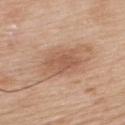This lesion was catalogued during total-body skin photography and was not selected for biopsy.
Automated tile analysis of the lesion measured a lesion area of about 9.5 mm², a shape eccentricity near 0.7, and a shape-asymmetry score of about 0.2 (0 = symmetric). The analysis additionally found a lesion color around L≈56 a*≈21 b*≈31 in CIELAB, roughly 9 lightness units darker than nearby skin, and a normalized border contrast of about 6. It also reported a border-irregularity rating of about 2.5/10, a color-variation rating of about 3/10, and peripheral color asymmetry of about 1. The software also gave an automated nevus-likeness rating near 70 out of 100 and a detector confidence of about 100 out of 100 that the crop contains a lesion.
The patient is a male about 60 years old.
The tile uses white-light illumination.
A region of skin cropped from a whole-body photographic capture, roughly 15 mm wide.
The recorded lesion diameter is about 4 mm.
From the upper back.